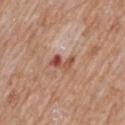notes — catalogued during a skin exam; not biopsied | size — ≈3 mm | patient — male, aged 58–62 | acquisition — ~15 mm tile from a whole-body skin photo | site — the mid back.